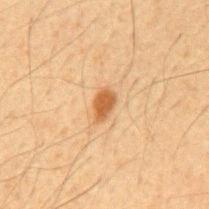workup=catalogued during a skin exam; not biopsied | location=the back | imaging modality=~15 mm tile from a whole-body skin photo | diameter=≈3 mm | patient=male, about 65 years old.